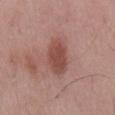Imaged during a routine full-body skin examination; the lesion was not biopsied and no histopathology is available. A 15 mm close-up tile from a total-body photography series done for melanoma screening. The subject is a male about 55 years old. From the mid back.